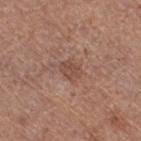Case summary:
– follow-up: catalogued during a skin exam; not biopsied
– location: the leg
– patient: female, aged 38 to 42
– automated lesion analysis: a mean CIELAB color near L≈48 a*≈21 b*≈27, a lesion–skin lightness drop of about 7, and a lesion-to-skin contrast of about 6 (normalized; higher = more distinct); a border-irregularity index near 2/10, a within-lesion color-variation index near 1.5/10, and a peripheral color-asymmetry measure near 0.5; lesion-presence confidence of about 100/100
– image source: ~15 mm tile from a whole-body skin photo
– diameter: ~2.5 mm (longest diameter)
– tile lighting: white-light